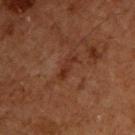Q: Is there a histopathology result?
A: imaged on a skin check; not biopsied
Q: What are the patient's age and sex?
A: male, aged 58–62
Q: Illumination type?
A: cross-polarized illumination
Q: What kind of image is this?
A: ~15 mm crop, total-body skin-cancer survey
Q: What is the lesion's diameter?
A: ≈3 mm
Q: What did automated image analysis measure?
A: a lesion area of about 3 mm², an eccentricity of roughly 0.9, and a symmetry-axis asymmetry near 0.3; a lesion color around L≈23 a*≈19 b*≈23 in CIELAB, about 5 CIELAB-L* units darker than the surrounding skin, and a normalized lesion–skin contrast near 6; a border-irregularity rating of about 3.5/10, a color-variation rating of about 1/10, and a peripheral color-asymmetry measure near 0
Q: What is the anatomic site?
A: the upper back